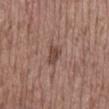biopsy_status: not biopsied; imaged during a skin examination
lesion_size:
  long_diameter_mm_approx: 3.5
lighting: white-light
image:
  source: total-body photography crop
  field_of_view_mm: 15
site: back
patient:
  sex: male
  age_approx: 70